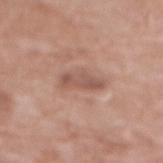Findings:
- patient — female, about 75 years old
- TBP lesion metrics — a classifier nevus-likeness of about 0/100 and a detector confidence of about 100 out of 100 that the crop contains a lesion
- tile lighting — white-light illumination
- site — the mid back
- imaging modality — ~15 mm crop, total-body skin-cancer survey